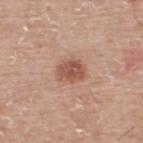The tile uses white-light illumination. About 3.5 mm across. A 15 mm close-up extracted from a 3D total-body photography capture. A male subject, in their mid- to late 70s. From the back.